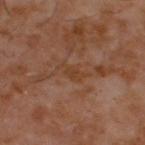Q: Was this lesion biopsied?
A: no biopsy performed (imaged during a skin exam)
Q: How was this image acquired?
A: ~15 mm tile from a whole-body skin photo
Q: What is the anatomic site?
A: the upper back
Q: Lesion size?
A: ~3.5 mm (longest diameter)
Q: What did automated image analysis measure?
A: a mean CIELAB color near L≈37 a*≈19 b*≈29 and roughly 5 lightness units darker than nearby skin; a peripheral color-asymmetry measure near 0.5
Q: What are the patient's age and sex?
A: male, approximately 60 years of age